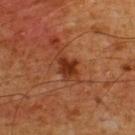Findings:
– follow-up: catalogued during a skin exam; not biopsied
– image: 15 mm crop, total-body photography
– body site: the upper back
– lighting: cross-polarized illumination
– lesion size: ~3.5 mm (longest diameter)
– patient: male, in their 60s
– image-analysis metrics: a lesion-detection confidence of about 100/100